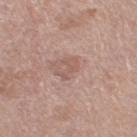Assessment:
Captured during whole-body skin photography for melanoma surveillance; the lesion was not biopsied.
Acquisition and patient details:
A 15 mm crop from a total-body photograph taken for skin-cancer surveillance. Captured under white-light illumination. The lesion is located on the right thigh. Approximately 3 mm at its widest. The subject is a female roughly 55 years of age.